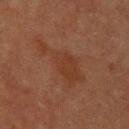{
  "biopsy_status": "not biopsied; imaged during a skin examination",
  "automated_metrics": {
    "eccentricity": 0.9,
    "shape_asymmetry": 0.5,
    "border_irregularity_0_10": 7.0,
    "color_variation_0_10": 2.0,
    "nevus_likeness_0_100": 0,
    "lesion_detection_confidence_0_100": 100
  },
  "image": {
    "source": "total-body photography crop",
    "field_of_view_mm": 15
  },
  "lesion_size": {
    "long_diameter_mm_approx": 7.5
  },
  "patient": {
    "sex": "male",
    "age_approx": 60
  },
  "site": "upper back"
}